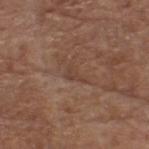Recorded during total-body skin imaging; not selected for excision or biopsy. This is a white-light tile. A 15 mm crop from a total-body photograph taken for skin-cancer surveillance. A male subject, in their 80s. From the head or neck.